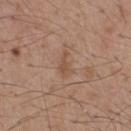Notes:
– workup: catalogued during a skin exam; not biopsied
– location: the upper back
– patient: male, aged approximately 50
– image: 15 mm crop, total-body photography
– lesion size: ≈3 mm
– lighting: white-light illumination
– TBP lesion metrics: an area of roughly 3.5 mm² and a symmetry-axis asymmetry near 0.45; a border-irregularity index near 4.5/10, a color-variation rating of about 1/10, and radial color variation of about 0.5; a classifier nevus-likeness of about 0/100 and lesion-presence confidence of about 100/100